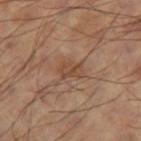Clinical impression: This lesion was catalogued during total-body skin photography and was not selected for biopsy. Context: The lesion is located on the leg. An algorithmic analysis of the crop reported a footprint of about 4.5 mm² and a shape eccentricity near 0.75. The software also gave an automated nevus-likeness rating near 0 out of 100 and a lesion-detection confidence of about 100/100. A male subject aged around 60. A 15 mm crop from a total-body photograph taken for skin-cancer surveillance.